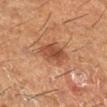Impression:
Part of a total-body skin-imaging series; this lesion was reviewed on a skin check and was not flagged for biopsy.
Background:
Cropped from a whole-body photographic skin survey; the tile spans about 15 mm. A female patient aged approximately 50. An algorithmic analysis of the crop reported an area of roughly 8.5 mm², an eccentricity of roughly 0.6, and two-axis asymmetry of about 0.15. It also reported a lesion color around L≈39 a*≈21 b*≈28 in CIELAB, a lesion–skin lightness drop of about 9, and a normalized border contrast of about 7.5. It also reported border irregularity of about 2 on a 0–10 scale. The software also gave a nevus-likeness score of about 70/100. About 3.5 mm across. From the right lower leg.